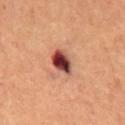The lesion was photographed on a routine skin check and not biopsied; there is no pathology result. This image is a 15 mm lesion crop taken from a total-body photograph. The tile uses cross-polarized illumination. The lesion is located on the chest. The patient is a male aged 63 to 67. About 3.5 mm across. Automated image analysis of the tile measured an area of roughly 6 mm² and two-axis asymmetry of about 0.2. It also reported a detector confidence of about 100 out of 100 that the crop contains a lesion.